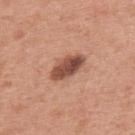Assessment: Part of a total-body skin-imaging series; this lesion was reviewed on a skin check and was not flagged for biopsy. Background: The lesion is located on the back. A close-up tile cropped from a whole-body skin photograph, about 15 mm across. The patient is a male approximately 55 years of age.